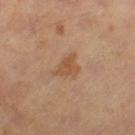The lesion was tiled from a total-body skin photograph and was not biopsied. Automated tile analysis of the lesion measured a border-irregularity index near 4/10 and radial color variation of about 1. And it measured a detector confidence of about 100 out of 100 that the crop contains a lesion. Longest diameter approximately 3 mm. The lesion is on the left thigh. A roughly 15 mm field-of-view crop from a total-body skin photograph. Imaged with cross-polarized lighting. The patient is a male aged 63 to 67.